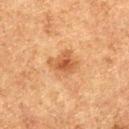The lesion was tiled from a total-body skin photograph and was not biopsied. The patient is a male in their mid- to late 70s. Cropped from a total-body skin-imaging series; the visible field is about 15 mm. From the left thigh. The tile uses cross-polarized illumination.